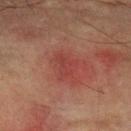Acquisition and patient details:
A male subject, about 75 years old. Cropped from a total-body skin-imaging series; the visible field is about 15 mm. On the left forearm.